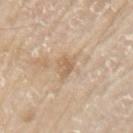- notes — catalogued during a skin exam; not biopsied
- lesion diameter — ≈2.5 mm
- acquisition — 15 mm crop, total-body photography
- lighting — white-light illumination
- location — the arm
- patient — male, approximately 80 years of age